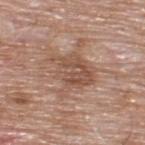The lesion was tiled from a total-body skin photograph and was not biopsied. Approximately 5 mm at its widest. Located on the upper back. This is a white-light tile. A region of skin cropped from a whole-body photographic capture, roughly 15 mm wide. The subject is a male approximately 60 years of age.